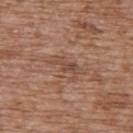On the back. The subject is a female aged 73 to 77. Cropped from a whole-body photographic skin survey; the tile spans about 15 mm. The lesion-visualizer software estimated a normalized lesion–skin contrast near 6. It also reported an automated nevus-likeness rating near 0 out of 100. Captured under white-light illumination. The lesion's longest dimension is about 3.5 mm.